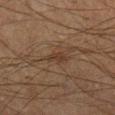Imaged during a routine full-body skin examination; the lesion was not biopsied and no histopathology is available.
Approximately 3 mm at its widest.
The tile uses cross-polarized illumination.
A lesion tile, about 15 mm wide, cut from a 3D total-body photograph.
The lesion is located on the right lower leg.
A male subject, in their mid-60s.